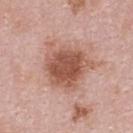Recorded during total-body skin imaging; not selected for excision or biopsy.
A lesion tile, about 15 mm wide, cut from a 3D total-body photograph.
From the upper back.
A female patient aged 28 to 32.